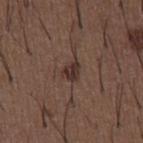biopsy_status: not biopsied; imaged during a skin examination
lighting: white-light
lesion_size:
  long_diameter_mm_approx: 2.5
site: front of the torso
patient:
  sex: male
  age_approx: 50
image:
  source: total-body photography crop
  field_of_view_mm: 15